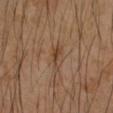Q: Lesion size?
A: ≈3.5 mm
Q: Automated lesion metrics?
A: an area of roughly 3.5 mm²; a classifier nevus-likeness of about 25/100 and a lesion-detection confidence of about 90/100
Q: Patient demographics?
A: female, aged 43 to 47
Q: What kind of image is this?
A: 15 mm crop, total-body photography
Q: Where on the body is the lesion?
A: the left forearm
Q: Illumination type?
A: cross-polarized